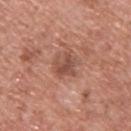Recorded during total-body skin imaging; not selected for excision or biopsy. A male subject in their mid-50s. The lesion-visualizer software estimated an area of roughly 6.5 mm², a shape eccentricity near 0.45, and a shape-asymmetry score of about 0.15 (0 = symmetric). The analysis additionally found a lesion color around L≈50 a*≈23 b*≈28 in CIELAB, roughly 9 lightness units darker than nearby skin, and a normalized lesion–skin contrast near 7. The analysis additionally found a border-irregularity index near 2.5/10, internal color variation of about 4 on a 0–10 scale, and peripheral color asymmetry of about 1.5. On the back. A lesion tile, about 15 mm wide, cut from a 3D total-body photograph.